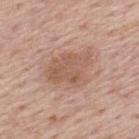follow-up: catalogued during a skin exam; not biopsied | subject: male, about 75 years old | automated lesion analysis: roughly 9 lightness units darker than nearby skin and a lesion-to-skin contrast of about 6.5 (normalized; higher = more distinct); a border-irregularity rating of about 3.5/10, a color-variation rating of about 4/10, and peripheral color asymmetry of about 1.5 | illumination: white-light illumination | imaging modality: 15 mm crop, total-body photography | location: the back.